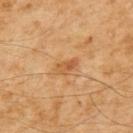biopsy status: imaged on a skin check; not biopsied | size: about 3 mm | imaging modality: total-body-photography crop, ~15 mm field of view | anatomic site: the upper back | image-analysis metrics: an outline eccentricity of about 0.85 (0 = round, 1 = elongated); a lesion color around L≈51 a*≈22 b*≈38 in CIELAB, a lesion–skin lightness drop of about 8, and a lesion-to-skin contrast of about 6 (normalized; higher = more distinct); a classifier nevus-likeness of about 5/100 and a detector confidence of about 100 out of 100 that the crop contains a lesion | tile lighting: cross-polarized | subject: male, aged around 60.